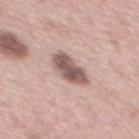Part of a total-body skin-imaging series; this lesion was reviewed on a skin check and was not flagged for biopsy.
A region of skin cropped from a whole-body photographic capture, roughly 15 mm wide.
Captured under white-light illumination.
Approximately 5 mm at its widest.
The subject is a male aged 63–67.
On the mid back.